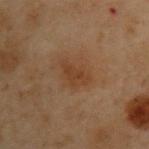Acquisition and patient details: A male patient aged approximately 55. A 15 mm close-up extracted from a 3D total-body photography capture. Captured under cross-polarized illumination. An algorithmic analysis of the crop reported a nevus-likeness score of about 10/100. The lesion's longest dimension is about 3.5 mm. Located on the upper back.